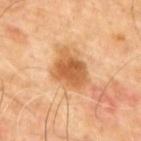Q: Was this lesion biopsied?
A: catalogued during a skin exam; not biopsied
Q: How was this image acquired?
A: total-body-photography crop, ~15 mm field of view
Q: How was the tile lit?
A: cross-polarized illumination
Q: What is the lesion's diameter?
A: ~5 mm (longest diameter)
Q: Lesion location?
A: the back
Q: What are the patient's age and sex?
A: male, in their mid-60s